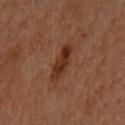• biopsy status · total-body-photography surveillance lesion; no biopsy
• patient · female, about 60 years old
• anatomic site · the right arm
• lesion size · ~4 mm (longest diameter)
• imaging modality · ~15 mm crop, total-body skin-cancer survey